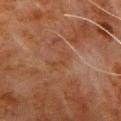Impression:
Recorded during total-body skin imaging; not selected for excision or biopsy.
Clinical summary:
On the front of the torso. A region of skin cropped from a whole-body photographic capture, roughly 15 mm wide. A male subject aged 78–82.